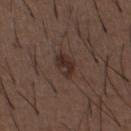{
  "biopsy_status": "not biopsied; imaged during a skin examination",
  "automated_metrics": {
    "peripheral_color_asymmetry": 1.5
  },
  "lesion_size": {
    "long_diameter_mm_approx": 3.0
  },
  "image": {
    "source": "total-body photography crop",
    "field_of_view_mm": 15
  },
  "lighting": "white-light",
  "site": "abdomen",
  "patient": {
    "sex": "male",
    "age_approx": 50
  }
}